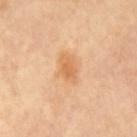Recorded during total-body skin imaging; not selected for excision or biopsy.
The lesion is located on the mid back.
Measured at roughly 3.5 mm in maximum diameter.
This is a cross-polarized tile.
Cropped from a total-body skin-imaging series; the visible field is about 15 mm.
Automated tile analysis of the lesion measured a lesion color around L≈68 a*≈23 b*≈42 in CIELAB, about 9 CIELAB-L* units darker than the surrounding skin, and a lesion-to-skin contrast of about 6 (normalized; higher = more distinct).